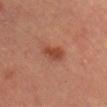Captured during whole-body skin photography for melanoma surveillance; the lesion was not biopsied.
An algorithmic analysis of the crop reported a lesion area of about 6 mm², an eccentricity of roughly 0.75, and two-axis asymmetry of about 0.25.
Approximately 3.5 mm at its widest.
From the head or neck.
A 15 mm close-up extracted from a 3D total-body photography capture.
A female patient in their mid-40s.
The tile uses cross-polarized illumination.